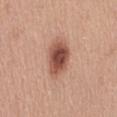Case summary:
• workup · catalogued during a skin exam; not biopsied
• body site · the abdomen
• size · ~4.5 mm (longest diameter)
• image source · ~15 mm tile from a whole-body skin photo
• tile lighting · white-light illumination
• subject · female, in their mid-50s
• automated metrics · an average lesion color of about L≈51 a*≈24 b*≈28 (CIELAB) and a lesion-to-skin contrast of about 10.5 (normalized; higher = more distinct)